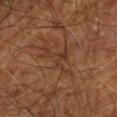Q: Was a biopsy performed?
A: imaged on a skin check; not biopsied
Q: Lesion location?
A: the left lower leg
Q: Illumination type?
A: cross-polarized
Q: Who is the patient?
A: approximately 65 years of age
Q: How was this image acquired?
A: total-body-photography crop, ~15 mm field of view
Q: What did automated image analysis measure?
A: an outline eccentricity of about 0.9 (0 = round, 1 = elongated) and a symmetry-axis asymmetry near 0.55; a classifier nevus-likeness of about 0/100 and a detector confidence of about 75 out of 100 that the crop contains a lesion
Q: Lesion size?
A: ~5.5 mm (longest diameter)